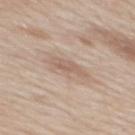An algorithmic analysis of the crop reported a mean CIELAB color near L≈60 a*≈16 b*≈26 and a lesion–skin lightness drop of about 8. The software also gave a border-irregularity index near 4/10, internal color variation of about 2.5 on a 0–10 scale, and a peripheral color-asymmetry measure near 0.5. Measured at roughly 4 mm in maximum diameter. The tile uses white-light illumination. This image is a 15 mm lesion crop taken from a total-body photograph. A male patient, in their 60s. The lesion is on the mid back.